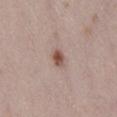Impression: Captured during whole-body skin photography for melanoma surveillance; the lesion was not biopsied. Acquisition and patient details: A roughly 15 mm field-of-view crop from a total-body skin photograph. A female patient, approximately 30 years of age. From the lower back.